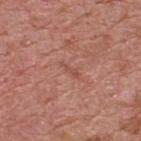Assessment: The lesion was tiled from a total-body skin photograph and was not biopsied. Clinical summary: From the upper back. A lesion tile, about 15 mm wide, cut from a 3D total-body photograph. A male subject, about 70 years old. Automated tile analysis of the lesion measured two-axis asymmetry of about 0.35. The analysis additionally found a border-irregularity index near 4/10, internal color variation of about 0 on a 0–10 scale, and radial color variation of about 0. And it measured a classifier nevus-likeness of about 0/100 and a detector confidence of about 95 out of 100 that the crop contains a lesion. Imaged with white-light lighting.